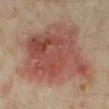Clinical impression:
Captured during whole-body skin photography for melanoma surveillance; the lesion was not biopsied.
Clinical summary:
The patient is a female aged approximately 35. The total-body-photography lesion software estimated an average lesion color of about L≈47 a*≈23 b*≈25 (CIELAB), roughly 11 lightness units darker than nearby skin, and a normalized border contrast of about 8. The tile uses cross-polarized illumination. Approximately 10.5 mm at its widest. The lesion is located on the right lower leg. This image is a 15 mm lesion crop taken from a total-body photograph.